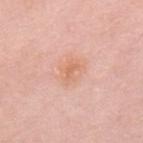- notes: no biopsy performed (imaged during a skin exam)
- image: 15 mm crop, total-body photography
- patient: male, in their mid-50s
- anatomic site: the mid back
- lighting: white-light illumination
- diameter: ≈3 mm